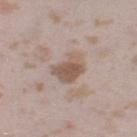Q: What did automated image analysis measure?
A: lesion-presence confidence of about 100/100
Q: How was the tile lit?
A: white-light
Q: Lesion size?
A: ≈3.5 mm
Q: What is the anatomic site?
A: the leg
Q: What kind of image is this?
A: 15 mm crop, total-body photography
Q: What are the patient's age and sex?
A: female, aged 23 to 27